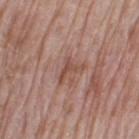No biopsy was performed on this lesion — it was imaged during a full skin examination and was not determined to be concerning. On the leg. A region of skin cropped from a whole-body photographic capture, roughly 15 mm wide. Imaged with white-light lighting. Measured at roughly 3.5 mm in maximum diameter. A female patient, about 75 years old.